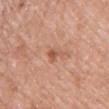Impression: No biopsy was performed on this lesion — it was imaged during a full skin examination and was not determined to be concerning. Context: The total-body-photography lesion software estimated a classifier nevus-likeness of about 5/100 and a detector confidence of about 100 out of 100 that the crop contains a lesion. Longest diameter approximately 3 mm. The lesion is on the chest. This image is a 15 mm lesion crop taken from a total-body photograph. A male patient about 50 years old. Captured under white-light illumination.